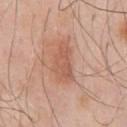The lesion was tiled from a total-body skin photograph and was not biopsied.
About 5 mm across.
Cropped from a total-body skin-imaging series; the visible field is about 15 mm.
A male patient roughly 55 years of age.
The lesion is located on the chest.